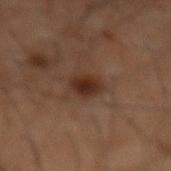Impression: Imaged during a routine full-body skin examination; the lesion was not biopsied and no histopathology is available. Image and clinical context: Captured under cross-polarized illumination. A male subject, roughly 60 years of age. The lesion is on the mid back. The recorded lesion diameter is about 3 mm. A 15 mm crop from a total-body photograph taken for skin-cancer surveillance.